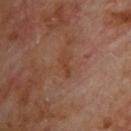This lesion was catalogued during total-body skin photography and was not selected for biopsy. The lesion is on the upper back. The tile uses cross-polarized illumination. A male subject, aged 68 to 72. Cropped from a total-body skin-imaging series; the visible field is about 15 mm.